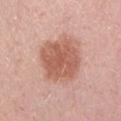<case>
<patient>
  <sex>male</sex>
  <age_approx>30</age_approx>
</patient>
<image>
  <source>total-body photography crop</source>
  <field_of_view_mm>15</field_of_view_mm>
</image>
</case>